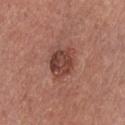Part of a total-body skin-imaging series; this lesion was reviewed on a skin check and was not flagged for biopsy. Cropped from a total-body skin-imaging series; the visible field is about 15 mm. The tile uses white-light illumination. The lesion is on the front of the torso. A male subject, aged around 35. An algorithmic analysis of the crop reported an outline eccentricity of about 0.5 (0 = round, 1 = elongated) and two-axis asymmetry of about 0.2. It also reported a color-variation rating of about 4/10.